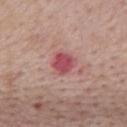workup = catalogued during a skin exam; not biopsied | imaging modality = ~15 mm crop, total-body skin-cancer survey | patient = male, in their mid- to late 70s | size = about 2.5 mm | tile lighting = white-light | location = the mid back.